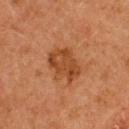Notes:
- notes · catalogued during a skin exam; not biopsied
- patient · female, in their 50s
- location · the upper back
- illumination · cross-polarized illumination
- size · about 4.5 mm
- imaging modality · ~15 mm crop, total-body skin-cancer survey
- TBP lesion metrics · a lesion area of about 11 mm², a shape eccentricity near 0.75, and a shape-asymmetry score of about 0.25 (0 = symmetric)